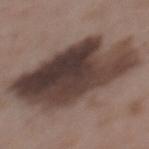| feature | finding |
|---|---|
| notes | total-body-photography surveillance lesion; no biopsy |
| patient | male, aged around 50 |
| lighting | white-light illumination |
| lesion size | ≈11 mm |
| anatomic site | the mid back |
| TBP lesion metrics | a lesion color around L≈38 a*≈14 b*≈19 in CIELAB and a lesion–skin lightness drop of about 16 |
| image | ~15 mm crop, total-body skin-cancer survey |